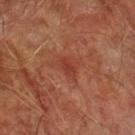Notes:
- follow-up — catalogued during a skin exam; not biopsied
- imaging modality — ~15 mm crop, total-body skin-cancer survey
- automated lesion analysis — a lesion area of about 3 mm² and a symmetry-axis asymmetry near 0.2; border irregularity of about 2.5 on a 0–10 scale, a color-variation rating of about 1.5/10, and peripheral color asymmetry of about 0.5
- body site — the left forearm
- patient — male, in their mid- to late 70s
- lesion size — ~2.5 mm (longest diameter)
- tile lighting — cross-polarized illumination